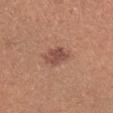| field | value |
|---|---|
| illumination | white-light |
| location | the arm |
| imaging modality | ~15 mm tile from a whole-body skin photo |
| patient | male, roughly 35 years of age |
| lesion diameter | about 3 mm |